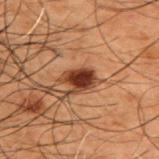workup — no biopsy performed (imaged during a skin exam)
image source — ~15 mm crop, total-body skin-cancer survey
subject — male, aged approximately 50
body site — the upper back